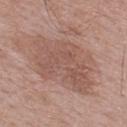biopsy status: total-body-photography surveillance lesion; no biopsy | location: the upper back | patient: male, approximately 70 years of age | lighting: white-light illumination | TBP lesion metrics: a lesion color around L≈54 a*≈20 b*≈25 in CIELAB and a lesion-to-skin contrast of about 5.5 (normalized; higher = more distinct); a border-irregularity rating of about 5.5/10, a color-variation rating of about 3/10, and peripheral color asymmetry of about 1 | image: 15 mm crop, total-body photography.